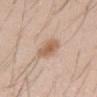biopsy status: imaged on a skin check; not biopsied | image source: 15 mm crop, total-body photography | body site: the abdomen | image-analysis metrics: a footprint of about 7.5 mm², an eccentricity of roughly 0.7, and two-axis asymmetry of about 0.15; an automated nevus-likeness rating near 85 out of 100 and lesion-presence confidence of about 100/100 | subject: male, aged 28–32 | diameter: ≈3.5 mm | tile lighting: white-light illumination.